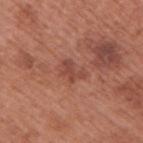The lesion was photographed on a routine skin check and not biopsied; there is no pathology result. The patient is a male about 70 years old. From the right upper arm. Cropped from a total-body skin-imaging series; the visible field is about 15 mm. This is a white-light tile. The total-body-photography lesion software estimated an area of roughly 3.5 mm², an eccentricity of roughly 0.75, and two-axis asymmetry of about 0.55. The analysis additionally found a border-irregularity index near 7/10 and peripheral color asymmetry of about 0.